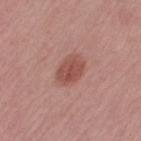workup: imaged on a skin check; not biopsied | imaging modality: ~15 mm tile from a whole-body skin photo | lighting: white-light illumination | lesion size: ~3.5 mm (longest diameter) | patient: female, in their mid- to late 60s | site: the right thigh.